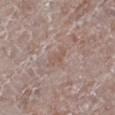Notes:
• follow-up · imaged on a skin check; not biopsied
• lighting · white-light
• lesion size · ≈3 mm
• body site · the right leg
• subject · male, aged approximately 70
• imaging modality · total-body-photography crop, ~15 mm field of view